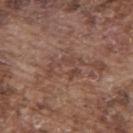image source: 15 mm crop, total-body photography
body site: the back
patient: male, aged approximately 75
automated lesion analysis: a footprint of about 7 mm² and two-axis asymmetry of about 0.7; a color-variation rating of about 1/10 and a peripheral color-asymmetry measure near 0; a nevus-likeness score of about 0/100
tile lighting: white-light